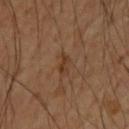A 15 mm close-up tile from a total-body photography series done for melanoma screening. Longest diameter approximately 3 mm. The patient is a male about 55 years old. From the back.